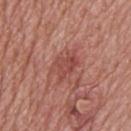Impression:
Recorded during total-body skin imaging; not selected for excision or biopsy.
Context:
Captured under white-light illumination. A roughly 15 mm field-of-view crop from a total-body skin photograph. The lesion is on the upper back. The total-body-photography lesion software estimated a footprint of about 8 mm², an eccentricity of roughly 0.55, and a symmetry-axis asymmetry near 0.25. The software also gave a lesion color around L≈48 a*≈27 b*≈26 in CIELAB, a lesion–skin lightness drop of about 8, and a lesion-to-skin contrast of about 6 (normalized; higher = more distinct). The lesion's longest dimension is about 4 mm. The patient is a male about 75 years old.